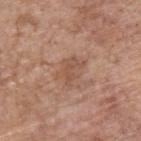Recorded during total-body skin imaging; not selected for excision or biopsy.